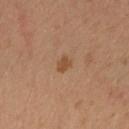| feature | finding |
|---|---|
| biopsy status | total-body-photography surveillance lesion; no biopsy |
| lighting | cross-polarized illumination |
| subject | female, aged 33–37 |
| location | the left forearm |
| image source | ~15 mm crop, total-body skin-cancer survey |